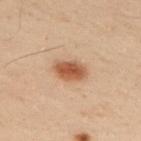biopsy_status: not biopsied; imaged during a skin examination
patient:
  sex: male
  age_approx: 50
lighting: cross-polarized
automated_metrics:
  area_mm2_approx: 7.0
  shape_asymmetry: 0.25
site: upper back
image:
  source: total-body photography crop
  field_of_view_mm: 15
lesion_size:
  long_diameter_mm_approx: 3.5